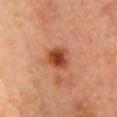follow-up: catalogued during a skin exam; not biopsied | subject: female, aged 33–37 | image source: ~15 mm crop, total-body skin-cancer survey | anatomic site: the front of the torso | tile lighting: cross-polarized illumination | size: about 3 mm.